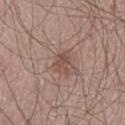workup = total-body-photography surveillance lesion; no biopsy
subject = male, approximately 65 years of age
lesion size = ~2.5 mm (longest diameter)
body site = the left thigh
tile lighting = white-light
acquisition = ~15 mm tile from a whole-body skin photo